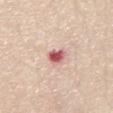biopsy status = no biopsy performed (imaged during a skin exam)
TBP lesion metrics = a shape eccentricity near 0.6 and two-axis asymmetry of about 0.3; a mean CIELAB color near L≈59 a*≈31 b*≈23 and a normalized border contrast of about 10.5; a border-irregularity index near 2.5/10, a within-lesion color-variation index near 7.5/10, and radial color variation of about 2.5
patient = female, about 55 years old
acquisition = ~15 mm tile from a whole-body skin photo
site = the chest
lesion diameter = ~2.5 mm (longest diameter)
tile lighting = white-light illumination